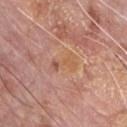Case summary:
- workup — no biopsy performed (imaged during a skin exam)
- patient — male, in their 60s
- automated metrics — a lesion area of about 5 mm² and a symmetry-axis asymmetry near 0.3; an average lesion color of about L≈57 a*≈22 b*≈33 (CIELAB) and a normalized lesion–skin contrast near 5
- anatomic site — the chest
- lighting — white-light illumination
- lesion diameter — about 3.5 mm
- image — 15 mm crop, total-body photography